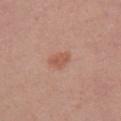{
  "image": {
    "source": "total-body photography crop",
    "field_of_view_mm": 15
  },
  "site": "chest",
  "lesion_size": {
    "long_diameter_mm_approx": 2.5
  },
  "patient": {
    "sex": "female",
    "age_approx": 25
  }
}